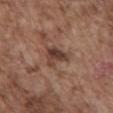Q: Is there a histopathology result?
A: total-body-photography surveillance lesion; no biopsy